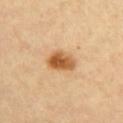Impression: Captured during whole-body skin photography for melanoma surveillance; the lesion was not biopsied. Clinical summary: This is a cross-polarized tile. Located on the chest. A female subject, about 40 years old. A 15 mm crop from a total-body photograph taken for skin-cancer surveillance. Measured at roughly 3.5 mm in maximum diameter. Automated image analysis of the tile measured an area of roughly 7 mm², an outline eccentricity of about 0.75 (0 = round, 1 = elongated), and a symmetry-axis asymmetry near 0.2. The software also gave a lesion–skin lightness drop of about 15. It also reported a classifier nevus-likeness of about 100/100 and a lesion-detection confidence of about 100/100.